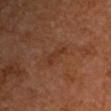{"biopsy_status": "not biopsied; imaged during a skin examination"}